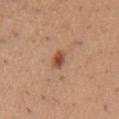<case>
  <biopsy_status>not biopsied; imaged during a skin examination</biopsy_status>
</case>